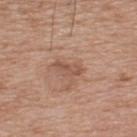Background:
A male subject, in their mid-60s. This is a white-light tile. Cropped from a total-body skin-imaging series; the visible field is about 15 mm. On the back.